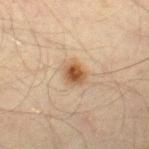notes: catalogued during a skin exam; not biopsied | subject: male, aged 43–47 | lighting: cross-polarized illumination | anatomic site: the right thigh | imaging modality: total-body-photography crop, ~15 mm field of view.